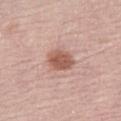Assessment:
The lesion was tiled from a total-body skin photograph and was not biopsied.
Image and clinical context:
A roughly 15 mm field-of-view crop from a total-body skin photograph. The lesion is on the abdomen. A female subject, in their mid-60s.